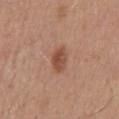  biopsy_status: not biopsied; imaged during a skin examination
  patient:
    sex: male
    age_approx: 55
  image:
    source: total-body photography crop
    field_of_view_mm: 15
  site: mid back
  lesion_size:
    long_diameter_mm_approx: 3.0
  automated_metrics:
    area_mm2_approx: 5.0
    eccentricity: 0.8
    shape_asymmetry: 0.2
    nevus_likeness_0_100: 95
    lesion_detection_confidence_0_100: 100
  lighting: white-light